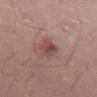Q: Was this lesion biopsied?
A: total-body-photography surveillance lesion; no biopsy
Q: What lighting was used for the tile?
A: white-light illumination
Q: What is the imaging modality?
A: ~15 mm crop, total-body skin-cancer survey
Q: Lesion location?
A: the right thigh
Q: What are the patient's age and sex?
A: female, aged 28–32